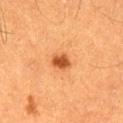Q: Was a biopsy performed?
A: no biopsy performed (imaged during a skin exam)
Q: Lesion size?
A: about 2.5 mm
Q: Where on the body is the lesion?
A: the left thigh
Q: What kind of image is this?
A: ~15 mm tile from a whole-body skin photo
Q: What are the patient's age and sex?
A: male, approximately 60 years of age